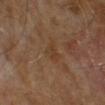* biopsy status — no biopsy performed (imaged during a skin exam)
* automated metrics — a lesion color around L≈37 a*≈16 b*≈30 in CIELAB, a lesion–skin lightness drop of about 4, and a normalized border contrast of about 4.5; a border-irregularity rating of about 3.5/10, internal color variation of about 2.5 on a 0–10 scale, and peripheral color asymmetry of about 1; lesion-presence confidence of about 100/100
* illumination — cross-polarized illumination
* subject — male, in their mid- to late 60s
* imaging modality — 15 mm crop, total-body photography
* diameter — about 2.5 mm